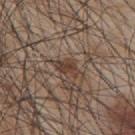{"biopsy_status": "not biopsied; imaged during a skin examination", "patient": {"sex": "male", "age_approx": 45}, "lighting": "white-light", "image": {"source": "total-body photography crop", "field_of_view_mm": 15}, "lesion_size": {"long_diameter_mm_approx": 3.0}, "site": "upper back", "automated_metrics": {"border_irregularity_0_10": 3.5, "color_variation_0_10": 2.0, "peripheral_color_asymmetry": 0.5, "nevus_likeness_0_100": 15, "lesion_detection_confidence_0_100": 80}}